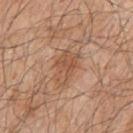No biopsy was performed on this lesion — it was imaged during a full skin examination and was not determined to be concerning.
The lesion is on the right upper arm.
A lesion tile, about 15 mm wide, cut from a 3D total-body photograph.
Automated image analysis of the tile measured an area of roughly 9 mm², an outline eccentricity of about 0.8 (0 = round, 1 = elongated), and a symmetry-axis asymmetry near 0.25. The analysis additionally found a color-variation rating of about 3.5/10. It also reported a lesion-detection confidence of about 100/100.
Longest diameter approximately 4.5 mm.
A male subject, aged around 80.